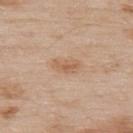The lesion is located on the upper back.
A male subject aged approximately 55.
Automated image analysis of the tile measured an outline eccentricity of about 0.85 (0 = round, 1 = elongated) and two-axis asymmetry of about 0.25. The analysis additionally found a lesion color around L≈60 a*≈19 b*≈33 in CIELAB, roughly 8 lightness units darker than nearby skin, and a lesion-to-skin contrast of about 6 (normalized; higher = more distinct).
Cropped from a total-body skin-imaging series; the visible field is about 15 mm.
The lesion's longest dimension is about 3.5 mm.
Captured under white-light illumination.